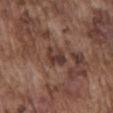The lesion was tiled from a total-body skin photograph and was not biopsied.